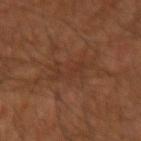workup = imaged on a skin check; not biopsied
site = the right forearm
patient = male, aged approximately 60
imaging modality = ~15 mm crop, total-body skin-cancer survey